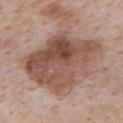Captured during whole-body skin photography for melanoma surveillance; the lesion was not biopsied.
Captured under white-light illumination.
A 15 mm close-up extracted from a 3D total-body photography capture.
From the back.
An algorithmic analysis of the crop reported a lesion area of about 46 mm² and a shape eccentricity near 0.75. The analysis additionally found an average lesion color of about L≈51 a*≈20 b*≈25 (CIELAB), a lesion–skin lightness drop of about 13, and a normalized lesion–skin contrast near 9. It also reported internal color variation of about 7.5 on a 0–10 scale and radial color variation of about 3.
A male patient aged approximately 75.
About 9.5 mm across.